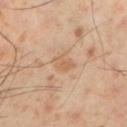Clinical impression: Imaged during a routine full-body skin examination; the lesion was not biopsied and no histopathology is available. Background: Captured under cross-polarized illumination. The lesion is on the left lower leg. A male patient, roughly 55 years of age. An algorithmic analysis of the crop reported an average lesion color of about L≈61 a*≈19 b*≈35 (CIELAB), about 7 CIELAB-L* units darker than the surrounding skin, and a normalized border contrast of about 6. Cropped from a whole-body photographic skin survey; the tile spans about 15 mm. The lesion's longest dimension is about 3 mm.